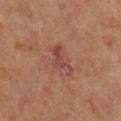<case>
  <biopsy_status>not biopsied; imaged during a skin examination</biopsy_status>
  <image>
    <source>total-body photography crop</source>
    <field_of_view_mm>15</field_of_view_mm>
  </image>
  <lesion_size>
    <long_diameter_mm_approx>4.5</long_diameter_mm_approx>
  </lesion_size>
  <patient>
    <sex>female</sex>
    <age_approx>65</age_approx>
  </patient>
  <site>right lower leg</site>
</case>